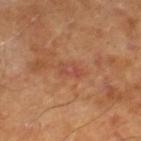<case>
  <biopsy_status>not biopsied; imaged during a skin examination</biopsy_status>
  <lighting>cross-polarized</lighting>
  <patient>
    <sex>male</sex>
    <age_approx>65</age_approx>
  </patient>
  <lesion_size>
    <long_diameter_mm_approx>3.0</long_diameter_mm_approx>
  </lesion_size>
  <automated_metrics>
    <area_mm2_approx>3.0</area_mm2_approx>
    <eccentricity>0.9</eccentricity>
    <shape_asymmetry>0.45</shape_asymmetry>
    <cielab_L>47</cielab_L>
    <cielab_a>26</cielab_a>
    <cielab_b>31</cielab_b>
    <vs_skin_darker_L>6.0</vs_skin_darker_L>
    <vs_skin_contrast_norm>5.0</vs_skin_contrast_norm>
    <border_irregularity_0_10>6.0</border_irregularity_0_10>
    <color_variation_0_10>0.0</color_variation_0_10>
    <peripheral_color_asymmetry>0.0</peripheral_color_asymmetry>
  </automated_metrics>
  <image>
    <source>total-body photography crop</source>
    <field_of_view_mm>15</field_of_view_mm>
  </image>
</case>